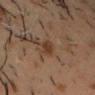A male subject, aged 48 to 52. A roughly 15 mm field-of-view crop from a total-body skin photograph. The total-body-photography lesion software estimated an area of roughly 4.5 mm², an eccentricity of roughly 0.6, and two-axis asymmetry of about 0.25. It also reported a border-irregularity rating of about 2/10 and internal color variation of about 1.5 on a 0–10 scale. This is a cross-polarized tile. The lesion is located on the front of the torso. Measured at roughly 2.5 mm in maximum diameter.